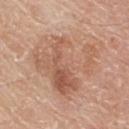This lesion was catalogued during total-body skin photography and was not selected for biopsy.
Automated image analysis of the tile measured an area of roughly 36 mm², a shape eccentricity near 0.65, and a symmetry-axis asymmetry near 0.5. It also reported a lesion color around L≈59 a*≈21 b*≈31 in CIELAB, a lesion–skin lightness drop of about 8, and a normalized lesion–skin contrast near 6.
The subject is a male aged around 80.
Imaged with white-light lighting.
A region of skin cropped from a whole-body photographic capture, roughly 15 mm wide.
From the upper back.